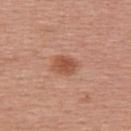Impression:
No biopsy was performed on this lesion — it was imaged during a full skin examination and was not determined to be concerning.
Acquisition and patient details:
On the upper back. This is a white-light tile. This image is a 15 mm lesion crop taken from a total-body photograph. The subject is a female aged around 55. The total-body-photography lesion software estimated a footprint of about 5.5 mm², a shape eccentricity near 0.7, and a shape-asymmetry score of about 0.15 (0 = symmetric). And it measured a border-irregularity rating of about 1.5/10, internal color variation of about 3 on a 0–10 scale, and a peripheral color-asymmetry measure near 1. And it measured a nevus-likeness score of about 95/100 and lesion-presence confidence of about 100/100. Measured at roughly 3 mm in maximum diameter.